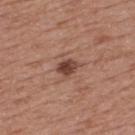| field | value |
|---|---|
| notes | catalogued during a skin exam; not biopsied |
| subject | male, aged 53–57 |
| anatomic site | the upper back |
| tile lighting | white-light illumination |
| automated lesion analysis | an automated nevus-likeness rating near 80 out of 100 |
| image source | ~15 mm crop, total-body skin-cancer survey |